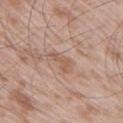Captured during whole-body skin photography for melanoma surveillance; the lesion was not biopsied.
This image is a 15 mm lesion crop taken from a total-body photograph.
The lesion is located on the right upper arm.
A male subject, aged 48–52.
Approximately 3 mm at its widest.
This is a white-light tile.